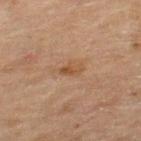Part of a total-body skin-imaging series; this lesion was reviewed on a skin check and was not flagged for biopsy. The lesion's longest dimension is about 3 mm. The total-body-photography lesion software estimated a shape eccentricity near 0.9 and a symmetry-axis asymmetry near 0.35. A close-up tile cropped from a whole-body skin photograph, about 15 mm across. A female subject aged 53 to 57. Captured under cross-polarized illumination. Located on the leg.